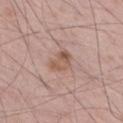Findings:
* notes: no biopsy performed (imaged during a skin exam)
* lighting: white-light
* image source: ~15 mm tile from a whole-body skin photo
* body site: the right thigh
* diameter: about 3.5 mm
* patient: male, aged approximately 55